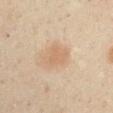notes: imaged on a skin check; not biopsied
body site: the right upper arm
automated metrics: an area of roughly 5.5 mm², an eccentricity of roughly 0.5, and a shape-asymmetry score of about 0.35 (0 = symmetric)
patient: male, approximately 50 years of age
tile lighting: cross-polarized illumination
diameter: ≈3 mm
image: ~15 mm tile from a whole-body skin photo